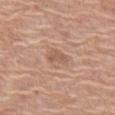A female subject, aged 58 to 62.
Longest diameter approximately 2.5 mm.
The lesion-visualizer software estimated an average lesion color of about L≈57 a*≈20 b*≈28 (CIELAB) and a normalized border contrast of about 5.5.
The lesion is on the leg.
This is a white-light tile.
Cropped from a total-body skin-imaging series; the visible field is about 15 mm.